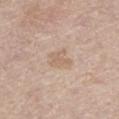* notes: imaged on a skin check; not biopsied
* acquisition: 15 mm crop, total-body photography
* anatomic site: the left thigh
* patient: male, aged 73–77
* illumination: white-light
* lesion diameter: ~3 mm (longest diameter)